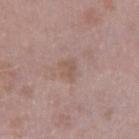<lesion>
<biopsy_status>not biopsied; imaged during a skin examination</biopsy_status>
<patient>
  <sex>male</sex>
  <age_approx>40</age_approx>
</patient>
<image>
  <source>total-body photography crop</source>
  <field_of_view_mm>15</field_of_view_mm>
</image>
<lesion_size>
  <long_diameter_mm_approx>2.5</long_diameter_mm_approx>
</lesion_size>
<site>right upper arm</site>
<automated_metrics>
  <cielab_L>54</cielab_L>
  <cielab_a>17</cielab_a>
  <cielab_b>25</cielab_b>
  <vs_skin_darker_L>7.0</vs_skin_darker_L>
  <vs_skin_contrast_norm>5.5</vs_skin_contrast_norm>
  <border_irregularity_0_10>4.0</border_irregularity_0_10>
  <nevus_likeness_0_100>0</nevus_likeness_0_100>
  <lesion_detection_confidence_0_100>100</lesion_detection_confidence_0_100>
</automated_metrics>
<lighting>white-light</lighting>
</lesion>